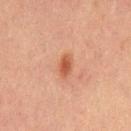The lesion was photographed on a routine skin check and not biopsied; there is no pathology result. The lesion is located on the mid back. About 3 mm across. A male patient, approximately 65 years of age. This image is a 15 mm lesion crop taken from a total-body photograph. Automated tile analysis of the lesion measured roughly 9 lightness units darker than nearby skin and a normalized border contrast of about 7.5. The tile uses cross-polarized illumination.